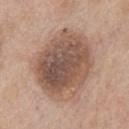notes: catalogued during a skin exam; not biopsied
image-analysis metrics: an area of roughly 40 mm², an eccentricity of roughly 0.6, and a symmetry-axis asymmetry near 0.15; a mean CIELAB color near L≈52 a*≈17 b*≈26, a lesion–skin lightness drop of about 14, and a lesion-to-skin contrast of about 9.5 (normalized; higher = more distinct); a within-lesion color-variation index near 6.5/10 and radial color variation of about 2; an automated nevus-likeness rating near 10 out of 100 and a lesion-detection confidence of about 100/100
location: the chest
image: 15 mm crop, total-body photography
subject: male, aged approximately 75
lesion size: about 8 mm
illumination: white-light illumination